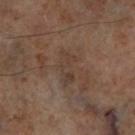Impression: Imaged during a routine full-body skin examination; the lesion was not biopsied and no histopathology is available. Clinical summary: Cropped from a whole-body photographic skin survey; the tile spans about 15 mm. This is a cross-polarized tile. The lesion is located on the leg.